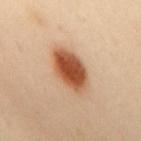Impression:
The lesion was photographed on a routine skin check and not biopsied; there is no pathology result.